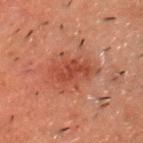Context:
This is a cross-polarized tile. Located on the chest. A region of skin cropped from a whole-body photographic capture, roughly 15 mm wide. The lesion-visualizer software estimated a classifier nevus-likeness of about 25/100 and a lesion-detection confidence of about 100/100. The lesion's longest dimension is about 5 mm. A male subject, roughly 50 years of age.